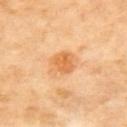  biopsy_status: not biopsied; imaged during a skin examination
  lesion_size:
    long_diameter_mm_approx: 3.0
  site: upper back
  image:
    source: total-body photography crop
    field_of_view_mm: 15
  lighting: cross-polarized
  automated_metrics:
    area_mm2_approx: 7.0
    eccentricity: 0.55
    shape_asymmetry: 0.1
    cielab_L: 69
    cielab_a: 26
    cielab_b: 47
    vs_skin_darker_L: 10.0
    border_irregularity_0_10: 1.0
    color_variation_0_10: 3.5
    peripheral_color_asymmetry: 1.0